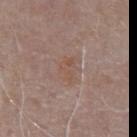This lesion was catalogued during total-body skin photography and was not selected for biopsy. Imaged with white-light lighting. The lesion's longest dimension is about 3 mm. Cropped from a total-body skin-imaging series; the visible field is about 15 mm. The subject is a male about 75 years old. On the chest.